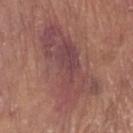biopsy status — total-body-photography surveillance lesion; no biopsy | illumination — white-light illumination | body site — the right upper arm | lesion diameter — ~10 mm (longest diameter) | patient — male, roughly 80 years of age | acquisition — total-body-photography crop, ~15 mm field of view | TBP lesion metrics — an average lesion color of about L≈46 a*≈23 b*≈20 (CIELAB), a lesion–skin lightness drop of about 8, and a lesion-to-skin contrast of about 7.5 (normalized; higher = more distinct); a color-variation rating of about 6/10 and peripheral color asymmetry of about 2; a classifier nevus-likeness of about 5/100 and a lesion-detection confidence of about 75/100.